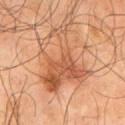biopsy status=catalogued during a skin exam; not biopsied